Imaged with white-light lighting; the lesion is located on the back; the patient is a male aged 53–57; about 7 mm across; a region of skin cropped from a whole-body photographic capture, roughly 15 mm wide:
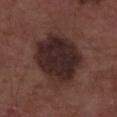On excision, pathology confirmed an atypical melanocytic neoplasm, classified as an indeterminate (borderline) lesion.